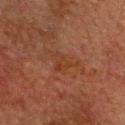Captured during whole-body skin photography for melanoma surveillance; the lesion was not biopsied.
About 4.5 mm across.
Located on the head or neck.
Imaged with cross-polarized lighting.
A male subject, aged around 60.
Cropped from a whole-body photographic skin survey; the tile spans about 15 mm.